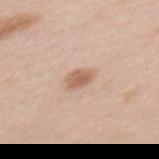Impression: Captured during whole-body skin photography for melanoma surveillance; the lesion was not biopsied. Image and clinical context: An algorithmic analysis of the crop reported an automated nevus-likeness rating near 85 out of 100. A roughly 15 mm field-of-view crop from a total-body skin photograph. About 2.5 mm across. This is a white-light tile. Located on the mid back. The subject is a female approximately 35 years of age.